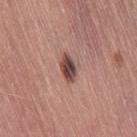Acquisition and patient details: On the leg. Cropped from a whole-body photographic skin survey; the tile spans about 15 mm. The patient is a female in their mid-60s.